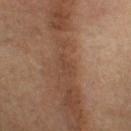Case summary:
• workup — imaged on a skin check; not biopsied
• illumination — cross-polarized
• anatomic site — the head or neck
• acquisition — 15 mm crop, total-body photography
• subject — female, aged approximately 65
• lesion diameter — about 2.5 mm